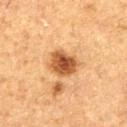Imaged during a routine full-body skin examination; the lesion was not biopsied and no histopathology is available.
On the left thigh.
A region of skin cropped from a whole-body photographic capture, roughly 15 mm wide.
Longest diameter approximately 4 mm.
The total-body-photography lesion software estimated an area of roughly 9.5 mm², an eccentricity of roughly 0.55, and a shape-asymmetry score of about 0.2 (0 = symmetric). The analysis additionally found roughly 14 lightness units darker than nearby skin. It also reported a nevus-likeness score of about 95/100 and a detector confidence of about 100 out of 100 that the crop contains a lesion.
The subject is a male about 75 years old.
Captured under cross-polarized illumination.